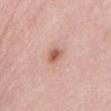Q: Was a biopsy performed?
A: imaged on a skin check; not biopsied
Q: What are the patient's age and sex?
A: female, aged around 70
Q: What kind of image is this?
A: total-body-photography crop, ~15 mm field of view
Q: Lesion location?
A: the front of the torso
Q: What is the lesion's diameter?
A: ~2.5 mm (longest diameter)